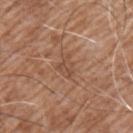Part of a total-body skin-imaging series; this lesion was reviewed on a skin check and was not flagged for biopsy.
A male subject, aged 73–77.
Cropped from a total-body skin-imaging series; the visible field is about 15 mm.
On the left upper arm.